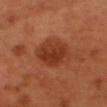The lesion was tiled from a total-body skin photograph and was not biopsied.